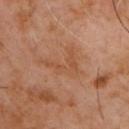Impression:
Recorded during total-body skin imaging; not selected for excision or biopsy.
Background:
The lesion is on the chest. A male subject aged 58–62. A 15 mm close-up tile from a total-body photography series done for melanoma screening. About 4.5 mm across.